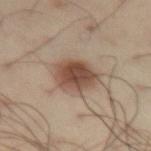No biopsy was performed on this lesion — it was imaged during a full skin examination and was not determined to be concerning.
The patient is a male aged 53 to 57.
This is a cross-polarized tile.
A lesion tile, about 15 mm wide, cut from a 3D total-body photograph.
From the abdomen.
Automated tile analysis of the lesion measured a footprint of about 13 mm² and an outline eccentricity of about 0.7 (0 = round, 1 = elongated). The analysis additionally found a lesion-detection confidence of about 100/100.
Longest diameter approximately 5 mm.